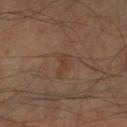Impression:
No biopsy was performed on this lesion — it was imaged during a full skin examination and was not determined to be concerning.
Context:
The lesion's longest dimension is about 2.5 mm. Automated image analysis of the tile measured border irregularity of about 4.5 on a 0–10 scale and a color-variation rating of about 1/10. And it measured a lesion-detection confidence of about 100/100. Cropped from a total-body skin-imaging series; the visible field is about 15 mm. A male patient aged approximately 70. Imaged with cross-polarized lighting. On the right forearm.